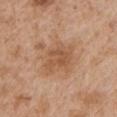Impression:
Recorded during total-body skin imaging; not selected for excision or biopsy.